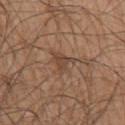Q: Was this lesion biopsied?
A: catalogued during a skin exam; not biopsied
Q: Lesion size?
A: ≈3 mm
Q: Automated lesion metrics?
A: an average lesion color of about L≈44 a*≈18 b*≈28 (CIELAB), about 8 CIELAB-L* units darker than the surrounding skin, and a normalized lesion–skin contrast near 6.5; internal color variation of about 2 on a 0–10 scale and a peripheral color-asymmetry measure near 0.5; a lesion-detection confidence of about 90/100
Q: What kind of image is this?
A: ~15 mm tile from a whole-body skin photo
Q: How was the tile lit?
A: white-light illumination
Q: Where on the body is the lesion?
A: the right upper arm
Q: Who is the patient?
A: male, in their mid- to late 70s